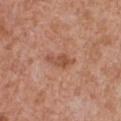<tbp_lesion>
<biopsy_status>not biopsied; imaged during a skin examination</biopsy_status>
<image>
  <source>total-body photography crop</source>
  <field_of_view_mm>15</field_of_view_mm>
</image>
<automated_metrics>
  <shape_asymmetry>0.35</shape_asymmetry>
  <cielab_L>51</cielab_L>
  <cielab_a>24</cielab_a>
  <cielab_b>32</cielab_b>
  <vs_skin_darker_L>9.0</vs_skin_darker_L>
  <border_irregularity_0_10>4.0</border_irregularity_0_10>
  <peripheral_color_asymmetry>1.0</peripheral_color_asymmetry>
  <nevus_likeness_0_100>10</nevus_likeness_0_100>
</automated_metrics>
<patient>
  <sex>male</sex>
  <age_approx>65</age_approx>
</patient>
<site>front of the torso</site>
<lesion_size>
  <long_diameter_mm_approx>3.5</long_diameter_mm_approx>
</lesion_size>
</tbp_lesion>